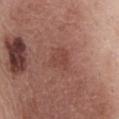{
  "biopsy_status": "not biopsied; imaged during a skin examination",
  "patient": {
    "sex": "female",
    "age_approx": 45
  },
  "image": {
    "source": "total-body photography crop",
    "field_of_view_mm": 15
  },
  "lighting": "white-light",
  "lesion_size": {
    "long_diameter_mm_approx": 3.0
  },
  "automated_metrics": {
    "cielab_L": 45,
    "cielab_a": 24,
    "cielab_b": 25,
    "vs_skin_darker_L": 6.0,
    "vs_skin_contrast_norm": 5.0
  },
  "site": "front of the torso"
}